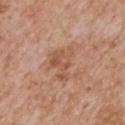Part of a total-body skin-imaging series; this lesion was reviewed on a skin check and was not flagged for biopsy.
This is a white-light tile.
A male subject in their mid-60s.
Measured at roughly 4 mm in maximum diameter.
A region of skin cropped from a whole-body photographic capture, roughly 15 mm wide.
The lesion is located on the mid back.